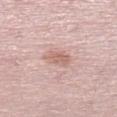Q: Was this lesion biopsied?
A: total-body-photography surveillance lesion; no biopsy
Q: What is the imaging modality?
A: ~15 mm tile from a whole-body skin photo
Q: Lesion size?
A: about 3 mm
Q: What is the anatomic site?
A: the leg
Q: Illumination type?
A: white-light illumination
Q: Who is the patient?
A: female, approximately 60 years of age